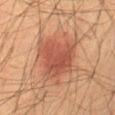Clinical impression: Part of a total-body skin-imaging series; this lesion was reviewed on a skin check and was not flagged for biopsy. Image and clinical context: Located on the abdomen. The subject is a male aged 58 to 62. This image is a 15 mm lesion crop taken from a total-body photograph.